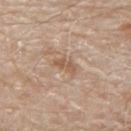The lesion was photographed on a routine skin check and not biopsied; there is no pathology result.
The subject is a male approximately 80 years of age.
The lesion-visualizer software estimated a mean CIELAB color near L≈57 a*≈17 b*≈31 and a lesion–skin lightness drop of about 8. The analysis additionally found border irregularity of about 3.5 on a 0–10 scale, a color-variation rating of about 2/10, and peripheral color asymmetry of about 0.5. And it measured a classifier nevus-likeness of about 0/100 and a detector confidence of about 100 out of 100 that the crop contains a lesion.
Longest diameter approximately 3 mm.
A 15 mm close-up extracted from a 3D total-body photography capture.
Imaged with white-light lighting.
From the back.